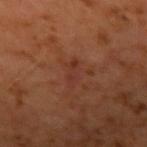workup: imaged on a skin check; not biopsied
lesion size: about 3 mm
patient: male, in their 60s
location: the left upper arm
imaging modality: ~15 mm tile from a whole-body skin photo
illumination: cross-polarized